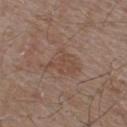Q: Was a biopsy performed?
A: no biopsy performed (imaged during a skin exam)
Q: How was this image acquired?
A: ~15 mm tile from a whole-body skin photo
Q: Lesion location?
A: the upper back
Q: Patient demographics?
A: male, aged 53–57